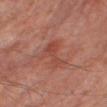Clinical impression:
The lesion was tiled from a total-body skin photograph and was not biopsied.
Image and clinical context:
A male patient, aged around 65. The tile uses cross-polarized illumination. An algorithmic analysis of the crop reported an average lesion color of about L≈44 a*≈26 b*≈27 (CIELAB), about 7 CIELAB-L* units darker than the surrounding skin, and a lesion-to-skin contrast of about 5.5 (normalized; higher = more distinct). Cropped from a total-body skin-imaging series; the visible field is about 15 mm. The recorded lesion diameter is about 4.5 mm. Located on the right thigh.